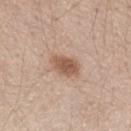{
  "biopsy_status": "not biopsied; imaged during a skin examination",
  "patient": {
    "sex": "female",
    "age_approx": 40
  },
  "image": {
    "source": "total-body photography crop",
    "field_of_view_mm": 15
  },
  "lesion_size": {
    "long_diameter_mm_approx": 3.5
  },
  "lighting": "white-light",
  "automated_metrics": {
    "area_mm2_approx": 7.0,
    "shape_asymmetry": 0.2
  },
  "site": "arm"
}